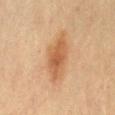biopsy status = total-body-photography surveillance lesion; no biopsy
patient = female, aged around 80
image = 15 mm crop, total-body photography
body site = the mid back
TBP lesion metrics = a border-irregularity index near 3/10, a color-variation rating of about 3.5/10, and peripheral color asymmetry of about 1; a nevus-likeness score of about 75/100
diameter = ≈6.5 mm
lighting = cross-polarized illumination